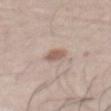This lesion was catalogued during total-body skin photography and was not selected for biopsy.
A 15 mm close-up tile from a total-body photography series done for melanoma screening.
A male subject, approximately 55 years of age.
The lesion is on the abdomen.